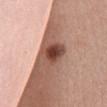The lesion was tiled from a total-body skin photograph and was not biopsied.